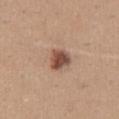biopsy status = catalogued during a skin exam; not biopsied | image source = ~15 mm crop, total-body skin-cancer survey | site = the mid back | lesion size = ≈3 mm | subject = female, aged 28–32 | automated lesion analysis = an area of roughly 6 mm², a shape eccentricity near 0.4, and two-axis asymmetry of about 0.25; a lesion color around L≈49 a*≈20 b*≈28 in CIELAB and about 15 CIELAB-L* units darker than the surrounding skin | illumination = white-light.